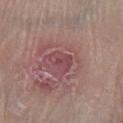Q: Is there a histopathology result?
A: catalogued during a skin exam; not biopsied
Q: Illumination type?
A: white-light
Q: What is the anatomic site?
A: the leg
Q: What kind of image is this?
A: ~15 mm crop, total-body skin-cancer survey
Q: What is the lesion's diameter?
A: ~3 mm (longest diameter)
Q: What did automated image analysis measure?
A: a footprint of about 6 mm² and a shape eccentricity near 0.6; a classifier nevus-likeness of about 0/100 and a detector confidence of about 60 out of 100 that the crop contains a lesion
Q: Patient demographics?
A: male, roughly 70 years of age